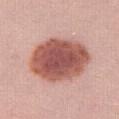{"biopsy_status": "not biopsied; imaged during a skin examination", "site": "right thigh", "patient": {"sex": "female", "age_approx": 25}, "lighting": "white-light", "image": {"source": "total-body photography crop", "field_of_view_mm": 15}, "automated_metrics": {"vs_skin_darker_L": 18.0, "vs_skin_contrast_norm": 11.5, "border_irregularity_0_10": 1.0, "color_variation_0_10": 5.0, "peripheral_color_asymmetry": 1.5, "nevus_likeness_0_100": 100, "lesion_detection_confidence_0_100": 100}, "lesion_size": {"long_diameter_mm_approx": 8.0}}